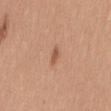Assessment: The lesion was tiled from a total-body skin photograph and was not biopsied. Context: On the mid back. The subject is a female aged around 35. Imaged with white-light lighting. Longest diameter approximately 2.5 mm. This image is a 15 mm lesion crop taken from a total-body photograph.